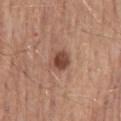notes: imaged on a skin check; not biopsied
body site: the mid back
imaging modality: ~15 mm tile from a whole-body skin photo
patient: male, aged approximately 55